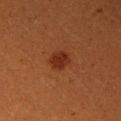<record>
<biopsy_status>not biopsied; imaged during a skin examination</biopsy_status>
<patient>
  <sex>female</sex>
  <age_approx>30</age_approx>
</patient>
<site>right upper arm</site>
<lighting>cross-polarized</lighting>
<lesion_size>
  <long_diameter_mm_approx>2.5</long_diameter_mm_approx>
</lesion_size>
<image>
  <source>total-body photography crop</source>
  <field_of_view_mm>15</field_of_view_mm>
</image>
</record>